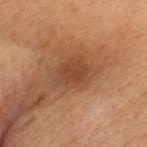Impression: Imaged during a routine full-body skin examination; the lesion was not biopsied and no histopathology is available. Clinical summary: Automated image analysis of the tile measured an average lesion color of about L≈39 a*≈20 b*≈30 (CIELAB) and a normalized border contrast of about 6.5. The analysis additionally found border irregularity of about 2.5 on a 0–10 scale, internal color variation of about 2.5 on a 0–10 scale, and a peripheral color-asymmetry measure near 1. It also reported a detector confidence of about 100 out of 100 that the crop contains a lesion. Located on the head or neck. The tile uses cross-polarized illumination. A female subject, aged 58–62. A region of skin cropped from a whole-body photographic capture, roughly 15 mm wide.